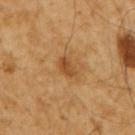notes — total-body-photography surveillance lesion; no biopsy | anatomic site — the arm | subject — male, in their 60s | image source — ~15 mm tile from a whole-body skin photo.